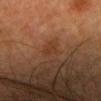Imaged during a routine full-body skin examination; the lesion was not biopsied and no histopathology is available.
A 15 mm close-up tile from a total-body photography series done for melanoma screening.
A female subject, aged 38 to 42.
Approximately 2.5 mm at its widest.
On the head or neck.
The total-body-photography lesion software estimated an outline eccentricity of about 0.8 (0 = round, 1 = elongated). The software also gave border irregularity of about 3.5 on a 0–10 scale, a within-lesion color-variation index near 1.5/10, and peripheral color asymmetry of about 0.5. The software also gave a nevus-likeness score of about 0/100 and a lesion-detection confidence of about 100/100.
The tile uses cross-polarized illumination.